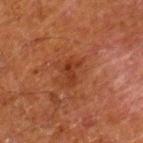Impression: Imaged during a routine full-body skin examination; the lesion was not biopsied and no histopathology is available. Background: The subject is a male approximately 80 years of age. A region of skin cropped from a whole-body photographic capture, roughly 15 mm wide. Captured under cross-polarized illumination. The lesion is located on the right lower leg.